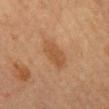Q: Was this lesion biopsied?
A: imaged on a skin check; not biopsied
Q: How was this image acquired?
A: total-body-photography crop, ~15 mm field of view
Q: Who is the patient?
A: female, aged approximately 60
Q: How large is the lesion?
A: ~5 mm (longest diameter)
Q: What lighting was used for the tile?
A: cross-polarized
Q: Lesion location?
A: the mid back